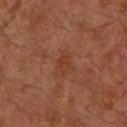workup: no biopsy performed (imaged during a skin exam); body site: the left upper arm; image source: 15 mm crop, total-body photography; subject: male, aged 28–32.